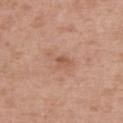Q: Is there a histopathology result?
A: total-body-photography surveillance lesion; no biopsy
Q: Who is the patient?
A: male, in their mid- to late 60s
Q: What lighting was used for the tile?
A: white-light
Q: Where on the body is the lesion?
A: the upper back
Q: What is the lesion's diameter?
A: ≈4 mm
Q: How was this image acquired?
A: ~15 mm tile from a whole-body skin photo